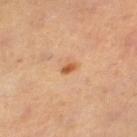Cropped from a total-body skin-imaging series; the visible field is about 15 mm. The lesion is located on the right thigh. A female subject, aged 38 to 42. Automated image analysis of the tile measured a detector confidence of about 100 out of 100 that the crop contains a lesion. Imaged with cross-polarized lighting.